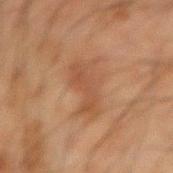This lesion was catalogued during total-body skin photography and was not selected for biopsy. This image is a 15 mm lesion crop taken from a total-body photograph. A male subject aged around 65. Automated tile analysis of the lesion measured a lesion color around L≈40 a*≈18 b*≈28 in CIELAB and about 6 CIELAB-L* units darker than the surrounding skin. And it measured a classifier nevus-likeness of about 0/100 and lesion-presence confidence of about 100/100. Approximately 5 mm at its widest. The lesion is located on the right forearm. The tile uses cross-polarized illumination.